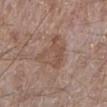The lesion was photographed on a routine skin check and not biopsied; there is no pathology result. A male patient about 55 years old. The lesion is located on the left lower leg. The recorded lesion diameter is about 4 mm. Captured under white-light illumination. This image is a 15 mm lesion crop taken from a total-body photograph.